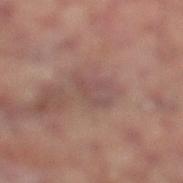On the left lower leg.
A 15 mm close-up extracted from a 3D total-body photography capture.
An algorithmic analysis of the crop reported a footprint of about 5.5 mm². The analysis additionally found roughly 5 lightness units darker than nearby skin and a normalized lesion–skin contrast near 4.5. It also reported a border-irregularity rating of about 6.5/10 and a color-variation rating of about 0.5/10. The software also gave a detector confidence of about 90 out of 100 that the crop contains a lesion.
Measured at roughly 3.5 mm in maximum diameter.
The tile uses cross-polarized illumination.
A male subject aged around 70.